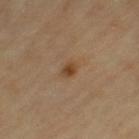workup — no biopsy performed (imaged during a skin exam)
subject — female, aged around 70
image — 15 mm crop, total-body photography
image-analysis metrics — internal color variation of about 4 on a 0–10 scale and radial color variation of about 1.5; a classifier nevus-likeness of about 80/100 and a detector confidence of about 100 out of 100 that the crop contains a lesion
tile lighting — cross-polarized illumination
lesion diameter — ≈2 mm
anatomic site — the left thigh